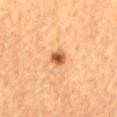Case summary:
- follow-up — imaged on a skin check; not biopsied
- image-analysis metrics — an eccentricity of roughly 0.55 and two-axis asymmetry of about 0.2; a lesion color around L≈52 a*≈25 b*≈39 in CIELAB and a lesion-to-skin contrast of about 10 (normalized; higher = more distinct)
- size — ≈2 mm
- patient — male, aged 53 to 57
- image source — 15 mm crop, total-body photography
- anatomic site — the mid back
- tile lighting — cross-polarized